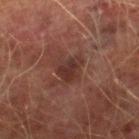Assessment:
Captured during whole-body skin photography for melanoma surveillance; the lesion was not biopsied.
Acquisition and patient details:
A 15 mm close-up tile from a total-body photography series done for melanoma screening. The lesion is located on the right lower leg. The subject is a male aged around 75.